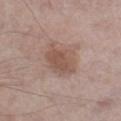| key | value |
|---|---|
| illumination | white-light |
| subject | male, roughly 55 years of age |
| location | the leg |
| acquisition | ~15 mm crop, total-body skin-cancer survey |